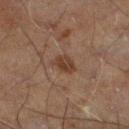follow-up: imaged on a skin check; not biopsied